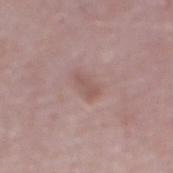Impression: The lesion was tiled from a total-body skin photograph and was not biopsied. Image and clinical context: From the abdomen. An algorithmic analysis of the crop reported a lesion area of about 3.5 mm² and two-axis asymmetry of about 0.3. It also reported a border-irregularity index near 3/10, a within-lesion color-variation index near 1/10, and radial color variation of about 0.5. The analysis additionally found a classifier nevus-likeness of about 0/100 and a lesion-detection confidence of about 100/100. A roughly 15 mm field-of-view crop from a total-body skin photograph. A male subject, in their mid- to late 70s. Longest diameter approximately 3 mm.